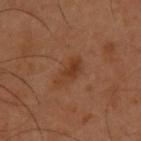Clinical impression: Imaged during a routine full-body skin examination; the lesion was not biopsied and no histopathology is available. Acquisition and patient details: Imaged with cross-polarized lighting. A 15 mm close-up extracted from a 3D total-body photography capture. Longest diameter approximately 3.5 mm. Located on the upper back. The patient is a male aged approximately 55.